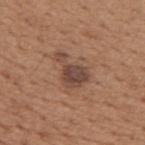Image and clinical context: The lesion is on the upper back. Imaged with white-light lighting. About 4.5 mm across. A male subject in their mid-60s. A roughly 15 mm field-of-view crop from a total-body skin photograph.